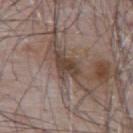subject=male, approximately 65 years of age
tile lighting=white-light
body site=the back
imaging modality=15 mm crop, total-body photography
size=about 4 mm